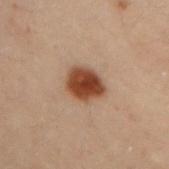workup: catalogued during a skin exam; not biopsied | lesion size: ~3.5 mm (longest diameter) | body site: the back | subject: male, roughly 30 years of age | image: ~15 mm tile from a whole-body skin photo | automated lesion analysis: a lesion area of about 9.5 mm², an outline eccentricity of about 0.5 (0 = round, 1 = elongated), and a symmetry-axis asymmetry near 0.15; a lesion color around L≈35 a*≈20 b*≈27 in CIELAB; a nevus-likeness score of about 100/100 and lesion-presence confidence of about 100/100.